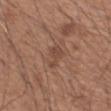Notes:
• illumination: white-light
• lesion diameter: ~3 mm (longest diameter)
• acquisition: 15 mm crop, total-body photography
• location: the right forearm
• patient: male, in their 50s
• automated lesion analysis: a lesion area of about 4.5 mm²; border irregularity of about 4.5 on a 0–10 scale, a color-variation rating of about 1/10, and peripheral color asymmetry of about 0.5; lesion-presence confidence of about 95/100